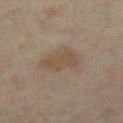Impression: The lesion was tiled from a total-body skin photograph and was not biopsied. Background: A female patient, aged around 35. From the left lower leg. A roughly 15 mm field-of-view crop from a total-body skin photograph. The lesion's longest dimension is about 3.5 mm.